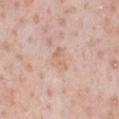workup — total-body-photography surveillance lesion; no biopsy
lesion size — ~3 mm (longest diameter)
subject — male, aged around 50
location — the chest
image source — ~15 mm tile from a whole-body skin photo